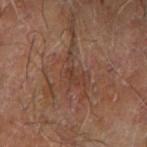notes: imaged on a skin check; not biopsied | image source: ~15 mm tile from a whole-body skin photo | lighting: cross-polarized illumination | site: the right forearm | patient: male, aged 63–67.